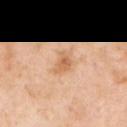{
  "biopsy_status": "not biopsied; imaged during a skin examination",
  "automated_metrics": {
    "color_variation_0_10": 4.0,
    "peripheral_color_asymmetry": 1.0,
    "nevus_likeness_0_100": 25,
    "lesion_detection_confidence_0_100": 100
  },
  "lesion_size": {
    "long_diameter_mm_approx": 3.0
  },
  "patient": {
    "sex": "male",
    "age_approx": 55
  },
  "lighting": "cross-polarized",
  "site": "left upper arm",
  "image": {
    "source": "total-body photography crop",
    "field_of_view_mm": 15
  }
}